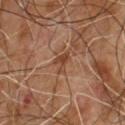<record>
<biopsy_status>not biopsied; imaged during a skin examination</biopsy_status>
<patient>
  <sex>male</sex>
  <age_approx>65</age_approx>
</patient>
<image>
  <source>total-body photography crop</source>
  <field_of_view_mm>15</field_of_view_mm>
</image>
</record>